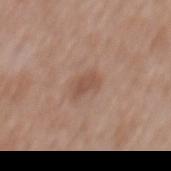Q: Was this lesion biopsied?
A: no biopsy performed (imaged during a skin exam)
Q: What did automated image analysis measure?
A: an area of roughly 4 mm², a shape eccentricity near 0.75, and a symmetry-axis asymmetry near 0.25
Q: How large is the lesion?
A: ≈2.5 mm
Q: What are the patient's age and sex?
A: male, in their 60s
Q: What lighting was used for the tile?
A: white-light
Q: What kind of image is this?
A: ~15 mm crop, total-body skin-cancer survey
Q: What is the anatomic site?
A: the mid back